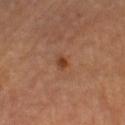The lesion-visualizer software estimated an area of roughly 2 mm², an outline eccentricity of about 0.65 (0 = round, 1 = elongated), and a shape-asymmetry score of about 0.35 (0 = symmetric). The analysis additionally found about 9 CIELAB-L* units darker than the surrounding skin and a normalized border contrast of about 8. And it measured a color-variation rating of about 1/10 and a peripheral color-asymmetry measure near 0.5. It also reported a detector confidence of about 100 out of 100 that the crop contains a lesion.
The patient is a female aged 63–67.
The lesion's longest dimension is about 2 mm.
Imaged with cross-polarized lighting.
From the left thigh.
A 15 mm crop from a total-body photograph taken for skin-cancer surveillance.